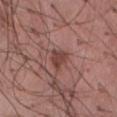Clinical impression: The lesion was tiled from a total-body skin photograph and was not biopsied. Acquisition and patient details: The total-body-photography lesion software estimated a mean CIELAB color near L≈43 a*≈23 b*≈23, a lesion–skin lightness drop of about 9, and a lesion-to-skin contrast of about 7.5 (normalized; higher = more distinct). The analysis additionally found a classifier nevus-likeness of about 80/100 and a detector confidence of about 100 out of 100 that the crop contains a lesion. Captured under white-light illumination. A male subject aged around 55. The lesion is on the abdomen. A 15 mm close-up tile from a total-body photography series done for melanoma screening. About 2.5 mm across.